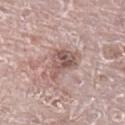Imaged during a routine full-body skin examination; the lesion was not biopsied and no histopathology is available. From the right leg. The patient is a male aged approximately 70. The tile uses white-light illumination. About 4 mm across. Cropped from a whole-body photographic skin survey; the tile spans about 15 mm.